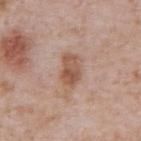illumination: white-light
image source: ~15 mm crop, total-body skin-cancer survey
subject: male, in their mid- to late 60s
lesion diameter: ≈3.5 mm
body site: the abdomen